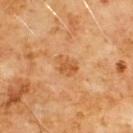No biopsy was performed on this lesion — it was imaged during a full skin examination and was not determined to be concerning. From the chest. This image is a 15 mm lesion crop taken from a total-body photograph. The patient is a male aged around 60. Captured under cross-polarized illumination.